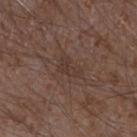About 3 mm across.
A close-up tile cropped from a whole-body skin photograph, about 15 mm across.
A male patient, aged around 75.
On the right forearm.
Imaged with white-light lighting.
Automated tile analysis of the lesion measured a footprint of about 4 mm², an eccentricity of roughly 0.8, and a symmetry-axis asymmetry near 0.4. The software also gave an average lesion color of about L≈35 a*≈16 b*≈22 (CIELAB), a lesion–skin lightness drop of about 5, and a normalized border contrast of about 5. And it measured a nevus-likeness score of about 0/100 and a lesion-detection confidence of about 100/100.